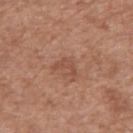Part of a total-body skin-imaging series; this lesion was reviewed on a skin check and was not flagged for biopsy. A male patient, aged approximately 55. A 15 mm close-up extracted from a 3D total-body photography capture. The lesion-visualizer software estimated a footprint of about 4 mm², a shape eccentricity near 0.8, and two-axis asymmetry of about 0.6. The software also gave an average lesion color of about L≈50 a*≈23 b*≈29 (CIELAB) and roughly 7 lightness units darker than nearby skin. The software also gave a border-irregularity index near 6.5/10, internal color variation of about 1 on a 0–10 scale, and peripheral color asymmetry of about 0.5. The software also gave a classifier nevus-likeness of about 0/100 and a lesion-detection confidence of about 100/100. This is a white-light tile. The lesion is on the right upper arm.